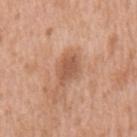biopsy_status: not biopsied; imaged during a skin examination
image:
  source: total-body photography crop
  field_of_view_mm: 15
site: mid back
patient:
  sex: male
  age_approx: 75
automated_metrics:
  area_mm2_approx: 6.5
  eccentricity: 0.75
  shape_asymmetry: 0.25
  cielab_L: 55
  cielab_a: 23
  cielab_b: 33
  vs_skin_darker_L: 10.0
  nevus_likeness_0_100: 35
  lesion_detection_confidence_0_100: 100